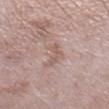A female patient roughly 70 years of age. The tile uses white-light illumination. Cropped from a total-body skin-imaging series; the visible field is about 15 mm. From the right lower leg. An algorithmic analysis of the crop reported an area of roughly 5 mm², an eccentricity of roughly 0.85, and a symmetry-axis asymmetry near 0.55. And it measured a lesion color around L≈58 a*≈17 b*≈23 in CIELAB, about 7 CIELAB-L* units darker than the surrounding skin, and a lesion-to-skin contrast of about 5.5 (normalized; higher = more distinct). And it measured border irregularity of about 6 on a 0–10 scale, a color-variation rating of about 1/10, and peripheral color asymmetry of about 0. The analysis additionally found a classifier nevus-likeness of about 0/100 and lesion-presence confidence of about 100/100. About 3.5 mm across.